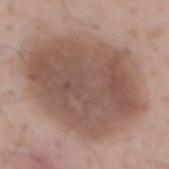{"biopsy_status": "not biopsied; imaged during a skin examination", "site": "mid back", "lighting": "white-light", "image": {"source": "total-body photography crop", "field_of_view_mm": 15}, "lesion_size": {"long_diameter_mm_approx": 12.0}, "patient": {"sex": "male", "age_approx": 55}}